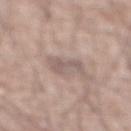notes: imaged on a skin check; not biopsied
acquisition: total-body-photography crop, ~15 mm field of view
lesion size: about 3 mm
tile lighting: white-light
subject: male, in their mid-70s
TBP lesion metrics: a lesion–skin lightness drop of about 8 and a lesion-to-skin contrast of about 6 (normalized; higher = more distinct); border irregularity of about 6 on a 0–10 scale, a within-lesion color-variation index near 0.5/10, and a peripheral color-asymmetry measure near 0; lesion-presence confidence of about 95/100
anatomic site: the abdomen